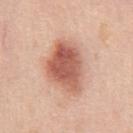Findings:
* notes · no biopsy performed (imaged during a skin exam)
* image-analysis metrics · an area of roughly 21 mm², an outline eccentricity of about 0.75 (0 = round, 1 = elongated), and a shape-asymmetry score of about 0.25 (0 = symmetric); border irregularity of about 2.5 on a 0–10 scale, a color-variation rating of about 6/10, and a peripheral color-asymmetry measure near 2; a nevus-likeness score of about 100/100 and a detector confidence of about 100 out of 100 that the crop contains a lesion
* illumination · white-light
* site · the chest
* lesion size · about 6.5 mm
* image source · total-body-photography crop, ~15 mm field of view
* patient · male, aged 48–52